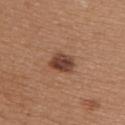Clinical impression:
Recorded during total-body skin imaging; not selected for excision or biopsy.
Context:
Located on the upper back. Automated tile analysis of the lesion measured a footprint of about 6.5 mm², an outline eccentricity of about 0.6 (0 = round, 1 = elongated), and a symmetry-axis asymmetry near 0.25. The analysis additionally found a lesion–skin lightness drop of about 13 and a lesion-to-skin contrast of about 10.5 (normalized; higher = more distinct). It also reported an automated nevus-likeness rating near 80 out of 100 and a detector confidence of about 100 out of 100 that the crop contains a lesion. Cropped from a whole-body photographic skin survey; the tile spans about 15 mm. The tile uses white-light illumination. The recorded lesion diameter is about 3 mm. A female subject, aged around 45.